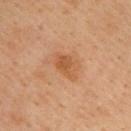The lesion was tiled from a total-body skin photograph and was not biopsied.
Measured at roughly 3 mm in maximum diameter.
Imaged with cross-polarized lighting.
Automated image analysis of the tile measured border irregularity of about 2.5 on a 0–10 scale and internal color variation of about 3 on a 0–10 scale. It also reported an automated nevus-likeness rating near 45 out of 100.
The lesion is located on the upper back.
This image is a 15 mm lesion crop taken from a total-body photograph.
The patient is a male aged 38–42.